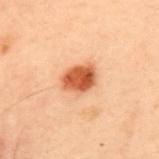Q: Was this lesion biopsied?
A: no biopsy performed (imaged during a skin exam)
Q: How large is the lesion?
A: about 4 mm
Q: What are the patient's age and sex?
A: male, in their mid- to late 50s
Q: Illumination type?
A: cross-polarized illumination
Q: What did automated image analysis measure?
A: a shape eccentricity near 0.65 and a shape-asymmetry score of about 0.2 (0 = symmetric)
Q: Lesion location?
A: the mid back
Q: How was this image acquired?
A: 15 mm crop, total-body photography